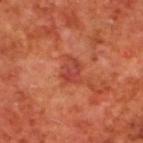Q: Is there a histopathology result?
A: total-body-photography surveillance lesion; no biopsy
Q: Where on the body is the lesion?
A: the upper back
Q: What are the patient's age and sex?
A: male, aged around 70
Q: What lighting was used for the tile?
A: cross-polarized illumination
Q: How was this image acquired?
A: ~15 mm crop, total-body skin-cancer survey
Q: How large is the lesion?
A: about 3 mm